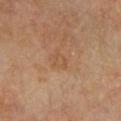| feature | finding |
|---|---|
| notes | catalogued during a skin exam; not biopsied |
| lighting | cross-polarized |
| image source | total-body-photography crop, ~15 mm field of view |
| automated lesion analysis | a lesion area of about 3 mm², an eccentricity of roughly 0.75, and two-axis asymmetry of about 0.35; a mean CIELAB color near L≈50 a*≈19 b*≈33, a lesion–skin lightness drop of about 4, and a normalized lesion–skin contrast near 4; a classifier nevus-likeness of about 0/100 and lesion-presence confidence of about 100/100 |
| anatomic site | the chest |
| patient | female, about 65 years old |